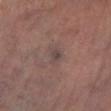biopsy_status: not biopsied; imaged during a skin examination
image:
  source: total-body photography crop
  field_of_view_mm: 15
site: left lower leg
lesion_size:
  long_diameter_mm_approx: 2.5
automated_metrics:
  area_mm2_approx: 3.5
  shape_asymmetry: 0.4
  border_irregularity_0_10: 3.5
  color_variation_0_10: 2.0
  peripheral_color_asymmetry: 0.5
patient:
  sex: male
  age_approx: 65